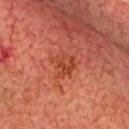biopsy status: no biopsy performed (imaged during a skin exam)
tile lighting: cross-polarized illumination
TBP lesion metrics: a lesion color around L≈35 a*≈27 b*≈29 in CIELAB, roughly 6 lightness units darker than nearby skin, and a lesion-to-skin contrast of about 6 (normalized; higher = more distinct); a border-irregularity rating of about 5/10 and a within-lesion color-variation index near 3/10
location: the head or neck
lesion diameter: about 3 mm
imaging modality: 15 mm crop, total-body photography
subject: male, aged around 80